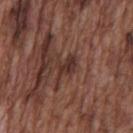Acquisition and patient details:
The recorded lesion diameter is about 3 mm. This is a white-light tile. The lesion is located on the chest. A lesion tile, about 15 mm wide, cut from a 3D total-body photograph. The patient is a male about 75 years old.